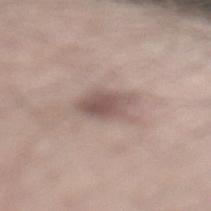Notes:
• notes — catalogued during a skin exam; not biopsied
• image — 15 mm crop, total-body photography
• patient — male, approximately 55 years of age
• diameter — ≈4.5 mm
• illumination — white-light illumination
• site — the leg